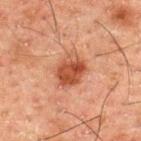Q: Was a biopsy performed?
A: catalogued during a skin exam; not biopsied
Q: How was the tile lit?
A: cross-polarized illumination
Q: What is the anatomic site?
A: the upper back
Q: What did automated image analysis measure?
A: an area of roughly 9 mm², an eccentricity of roughly 0.6, and a symmetry-axis asymmetry near 0.15; an average lesion color of about L≈39 a*≈24 b*≈29 (CIELAB) and about 11 CIELAB-L* units darker than the surrounding skin
Q: What is the imaging modality?
A: ~15 mm tile from a whole-body skin photo
Q: What are the patient's age and sex?
A: male, aged 48 to 52